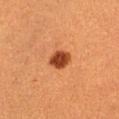workup=total-body-photography surveillance lesion; no biopsy | lighting=cross-polarized | lesion size=~2.5 mm (longest diameter) | acquisition=~15 mm tile from a whole-body skin photo | body site=the right thigh | automated lesion analysis=an area of roughly 5.5 mm² and an eccentricity of roughly 0.45; a classifier nevus-likeness of about 100/100 and lesion-presence confidence of about 100/100 | patient=female, roughly 55 years of age.